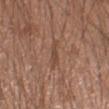This lesion was catalogued during total-body skin photography and was not selected for biopsy. On the right forearm. Cropped from a total-body skin-imaging series; the visible field is about 15 mm. The subject is a male aged approximately 20. Measured at roughly 3 mm in maximum diameter. Captured under white-light illumination.